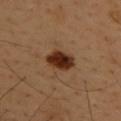<tbp_lesion>
<biopsy_status>not biopsied; imaged during a skin examination</biopsy_status>
<automated_metrics>
  <border_irregularity_0_10>1.5</border_irregularity_0_10>
  <color_variation_0_10>5.0</color_variation_0_10>
  <peripheral_color_asymmetry>1.5</peripheral_color_asymmetry>
  <nevus_likeness_0_100>100</nevus_likeness_0_100>
  <lesion_detection_confidence_0_100>100</lesion_detection_confidence_0_100>
</automated_metrics>
<image>
  <source>total-body photography crop</source>
  <field_of_view_mm>15</field_of_view_mm>
</image>
<site>upper back</site>
<lighting>cross-polarized</lighting>
<patient>
  <sex>male</sex>
  <age_approx>40</age_approx>
</patient>
</tbp_lesion>